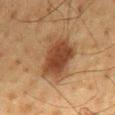No biopsy was performed on this lesion — it was imaged during a full skin examination and was not determined to be concerning. Measured at roughly 6 mm in maximum diameter. A male patient, about 60 years old. This image is a 15 mm lesion crop taken from a total-body photograph. Captured under cross-polarized illumination. The lesion is located on the back. Automated image analysis of the tile measured an average lesion color of about L≈35 a*≈17 b*≈27 (CIELAB), about 10 CIELAB-L* units darker than the surrounding skin, and a normalized border contrast of about 9.5. It also reported lesion-presence confidence of about 100/100.